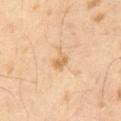Part of a total-body skin-imaging series; this lesion was reviewed on a skin check and was not flagged for biopsy.
The lesion-visualizer software estimated a border-irregularity rating of about 4/10, a within-lesion color-variation index near 2.5/10, and radial color variation of about 1.
The tile uses cross-polarized illumination.
A 15 mm close-up extracted from a 3D total-body photography capture.
About 3 mm across.
The subject is a male about 65 years old.